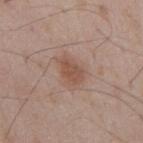Assessment:
Part of a total-body skin-imaging series; this lesion was reviewed on a skin check and was not flagged for biopsy.
Image and clinical context:
A male patient aged 48–52. A lesion tile, about 15 mm wide, cut from a 3D total-body photograph. The lesion is located on the mid back.